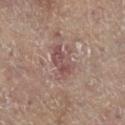  biopsy_status: not biopsied; imaged during a skin examination
  site: left leg
  lesion_size:
    long_diameter_mm_approx: 4.0
  patient:
    sex: female
    age_approx: 80
  automated_metrics:
    cielab_L: 44
    cielab_a: 18
    cielab_b: 19
    vs_skin_darker_L: 8.0
    nevus_likeness_0_100: 0
    lesion_detection_confidence_0_100: 95
  image:
    source: total-body photography crop
    field_of_view_mm: 15